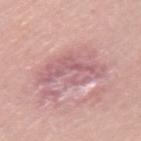The lesion is located on the arm. Measured at roughly 6.5 mm in maximum diameter. The total-body-photography lesion software estimated a border-irregularity index near 8.5/10, internal color variation of about 4 on a 0–10 scale, and a peripheral color-asymmetry measure near 1.5. The patient is a female approximately 35 years of age. Captured under white-light illumination. A 15 mm close-up extracted from a 3D total-body photography capture.